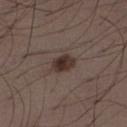Captured during whole-body skin photography for melanoma surveillance; the lesion was not biopsied.
Automated image analysis of the tile measured a shape eccentricity near 0.75. The analysis additionally found a mean CIELAB color near L≈32 a*≈14 b*≈19, roughly 11 lightness units darker than nearby skin, and a lesion-to-skin contrast of about 10.5 (normalized; higher = more distinct). The analysis additionally found border irregularity of about 1.5 on a 0–10 scale, internal color variation of about 4 on a 0–10 scale, and peripheral color asymmetry of about 1.5. The analysis additionally found a nevus-likeness score of about 95/100 and lesion-presence confidence of about 100/100.
A 15 mm close-up tile from a total-body photography series done for melanoma screening.
From the abdomen.
About 3 mm across.
The tile uses white-light illumination.
The patient is a male aged 48 to 52.